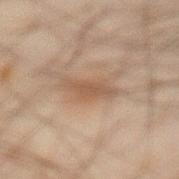The lesion was photographed on a routine skin check and not biopsied; there is no pathology result. Imaged with cross-polarized lighting. The recorded lesion diameter is about 4.5 mm. From the front of the torso. A male subject, aged approximately 45. A 15 mm close-up tile from a total-body photography series done for melanoma screening.